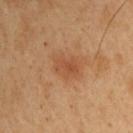biopsy status: imaged on a skin check; not biopsied
body site: the right upper arm
illumination: cross-polarized
patient: male, in their 50s
image source: ~15 mm tile from a whole-body skin photo